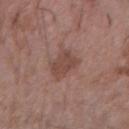The lesion was photographed on a routine skin check and not biopsied; there is no pathology result. A close-up tile cropped from a whole-body skin photograph, about 15 mm across. The patient is a male roughly 60 years of age. The lesion is on the right forearm.